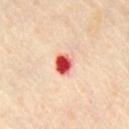No biopsy was performed on this lesion — it was imaged during a full skin examination and was not determined to be concerning.
The lesion is on the chest.
A female subject, aged 43–47.
The lesion-visualizer software estimated an average lesion color of about L≈56 a*≈42 b*≈33 (CIELAB) and about 23 CIELAB-L* units darker than the surrounding skin. And it measured a border-irregularity rating of about 2.5/10 and a color-variation rating of about 6.5/10. The software also gave a classifier nevus-likeness of about 0/100 and lesion-presence confidence of about 100/100.
A lesion tile, about 15 mm wide, cut from a 3D total-body photograph.